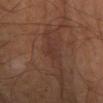- notes · total-body-photography surveillance lesion; no biopsy
- patient · male, approximately 65 years of age
- image source · total-body-photography crop, ~15 mm field of view
- size · about 2.5 mm
- anatomic site · the left lower leg
- illumination · cross-polarized illumination